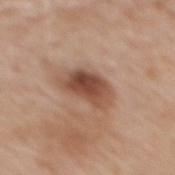notes: total-body-photography surveillance lesion; no biopsy
tile lighting: white-light illumination
image: ~15 mm tile from a whole-body skin photo
patient: male, approximately 70 years of age
lesion diameter: about 5 mm
site: the mid back